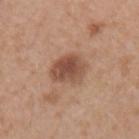Captured during whole-body skin photography for melanoma surveillance; the lesion was not biopsied. Cropped from a whole-body photographic skin survey; the tile spans about 15 mm. From the right forearm. The tile uses white-light illumination. The patient is a female in their 30s. Measured at roughly 4.5 mm in maximum diameter.